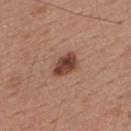Q: Was this lesion biopsied?
A: catalogued during a skin exam; not biopsied
Q: Illumination type?
A: white-light illumination
Q: What did automated image analysis measure?
A: an area of roughly 5.5 mm², an eccentricity of roughly 0.75, and a symmetry-axis asymmetry near 0.2; an average lesion color of about L≈42 a*≈22 b*≈27 (CIELAB) and a lesion-to-skin contrast of about 11.5 (normalized; higher = more distinct); a border-irregularity index near 2/10, a within-lesion color-variation index near 4/10, and radial color variation of about 1.5
Q: How was this image acquired?
A: 15 mm crop, total-body photography
Q: Who is the patient?
A: male, approximately 55 years of age
Q: Where on the body is the lesion?
A: the upper back